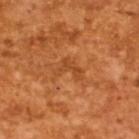Clinical summary:
A 15 mm crop from a total-body photograph taken for skin-cancer surveillance. Longest diameter approximately 3.5 mm. A male patient, aged 63 to 67. Automated tile analysis of the lesion measured a mean CIELAB color near L≈46 a*≈28 b*≈42, about 7 CIELAB-L* units darker than the surrounding skin, and a lesion-to-skin contrast of about 5 (normalized; higher = more distinct). The analysis additionally found a border-irregularity index near 7.5/10 and a within-lesion color-variation index near 0.5/10. The software also gave a nevus-likeness score of about 0/100 and a detector confidence of about 100 out of 100 that the crop contains a lesion.